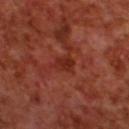Q: Is there a histopathology result?
A: catalogued during a skin exam; not biopsied
Q: Who is the patient?
A: male, aged approximately 70
Q: What is the imaging modality?
A: ~15 mm tile from a whole-body skin photo
Q: What is the anatomic site?
A: the upper back